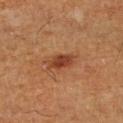Impression:
No biopsy was performed on this lesion — it was imaged during a full skin examination and was not determined to be concerning.
Background:
Measured at roughly 3.5 mm in maximum diameter. The lesion is located on the right lower leg. A male subject, aged 58 to 62. Cropped from a total-body skin-imaging series; the visible field is about 15 mm.